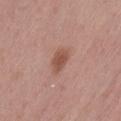{"biopsy_status": "not biopsied; imaged during a skin examination", "site": "back", "image": {"source": "total-body photography crop", "field_of_view_mm": 15}, "lesion_size": {"long_diameter_mm_approx": 3.0}, "lighting": "white-light", "patient": {"sex": "female", "age_approx": 60}, "automated_metrics": {"area_mm2_approx": 5.0, "shape_asymmetry": 0.25, "vs_skin_darker_L": 10.0, "vs_skin_contrast_norm": 7.5, "border_irregularity_0_10": 2.0, "color_variation_0_10": 2.0, "peripheral_color_asymmetry": 0.5, "nevus_likeness_0_100": 90, "lesion_detection_confidence_0_100": 100}}